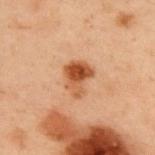biopsy_status: not biopsied; imaged during a skin examination
lesion_size:
  long_diameter_mm_approx: 3.5
site: upper back
lighting: cross-polarized
image:
  source: total-body photography crop
  field_of_view_mm: 15
patient:
  sex: male
  age_approx: 55